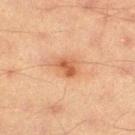Assessment:
Part of a total-body skin-imaging series; this lesion was reviewed on a skin check and was not flagged for biopsy.
Context:
Located on the right thigh. A roughly 15 mm field-of-view crop from a total-body skin photograph. A male patient, roughly 40 years of age. Imaged with cross-polarized lighting.